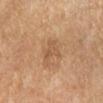<tbp_lesion>
  <biopsy_status>not biopsied; imaged during a skin examination</biopsy_status>
  <lesion_size>
    <long_diameter_mm_approx>2.5</long_diameter_mm_approx>
  </lesion_size>
  <automated_metrics>
    <border_irregularity_0_10>6.0</border_irregularity_0_10>
    <nevus_likeness_0_100>5</nevus_likeness_0_100>
    <lesion_detection_confidence_0_100>100</lesion_detection_confidence_0_100>
  </automated_metrics>
  <image>
    <source>total-body photography crop</source>
    <field_of_view_mm>15</field_of_view_mm>
  </image>
  <site>left lower leg</site>
  <patient>
    <sex>male</sex>
    <age_approx>65</age_approx>
  </patient>
</tbp_lesion>